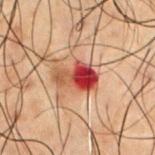Recorded during total-body skin imaging; not selected for excision or biopsy. The tile uses cross-polarized illumination. On the front of the torso. Approximately 4.5 mm at its widest. A male subject, about 50 years old. A lesion tile, about 15 mm wide, cut from a 3D total-body photograph.